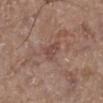Q: Was this lesion biopsied?
A: imaged on a skin check; not biopsied
Q: What kind of image is this?
A: total-body-photography crop, ~15 mm field of view
Q: Illumination type?
A: white-light illumination
Q: What is the anatomic site?
A: the left lower leg
Q: What did automated image analysis measure?
A: an area of roughly 3.5 mm², an eccentricity of roughly 0.8, and a shape-asymmetry score of about 0.3 (0 = symmetric); a mean CIELAB color near L≈46 a*≈20 b*≈24, a lesion–skin lightness drop of about 7, and a normalized lesion–skin contrast near 6
Q: What is the lesion's diameter?
A: ≈2.5 mm
Q: What are the patient's age and sex?
A: male, in their mid- to late 60s